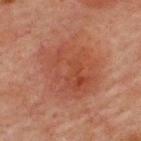Q: Is there a histopathology result?
A: total-body-photography surveillance lesion; no biopsy
Q: Lesion size?
A: ≈6.5 mm
Q: What lighting was used for the tile?
A: cross-polarized
Q: What are the patient's age and sex?
A: male, aged around 60
Q: What is the imaging modality?
A: total-body-photography crop, ~15 mm field of view
Q: Lesion location?
A: the upper back
Q: Automated lesion metrics?
A: a lesion area of about 33 mm², an eccentricity of roughly 0.45, and a shape-asymmetry score of about 0.2 (0 = symmetric); border irregularity of about 3 on a 0–10 scale, a within-lesion color-variation index near 4/10, and a peripheral color-asymmetry measure near 1.5